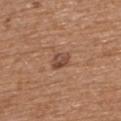{"biopsy_status": "not biopsied; imaged during a skin examination", "image": {"source": "total-body photography crop", "field_of_view_mm": 15}, "site": "back", "lesion_size": {"long_diameter_mm_approx": 2.5}, "patient": {"sex": "male", "age_approx": 70}}